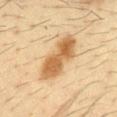Notes:
– biopsy status: catalogued during a skin exam; not biopsied
– lighting: cross-polarized illumination
– body site: the chest
– subject: male, roughly 35 years of age
– automated metrics: border irregularity of about 2.5 on a 0–10 scale, internal color variation of about 4 on a 0–10 scale, and peripheral color asymmetry of about 1.5; an automated nevus-likeness rating near 85 out of 100 and a lesion-detection confidence of about 100/100
– imaging modality: ~15 mm crop, total-body skin-cancer survey
– size: ~7 mm (longest diameter)